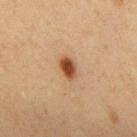This lesion was catalogued during total-body skin photography and was not selected for biopsy. Cropped from a whole-body photographic skin survey; the tile spans about 15 mm. The recorded lesion diameter is about 2.5 mm. Automated tile analysis of the lesion measured a lesion color around L≈40 a*≈20 b*≈32 in CIELAB and about 14 CIELAB-L* units darker than the surrounding skin. The lesion is on the back. Captured under cross-polarized illumination. A male subject aged approximately 60.